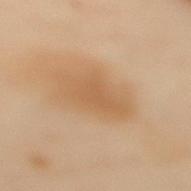Imaged during a routine full-body skin examination; the lesion was not biopsied and no histopathology is available.
The patient is a female aged around 60.
The lesion is located on the upper back.
A close-up tile cropped from a whole-body skin photograph, about 15 mm across.
Longest diameter approximately 5 mm.